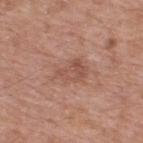Impression:
The lesion was photographed on a routine skin check and not biopsied; there is no pathology result.
Image and clinical context:
A male subject, aged 53–57. An algorithmic analysis of the crop reported a mean CIELAB color near L≈53 a*≈21 b*≈28 and a lesion–skin lightness drop of about 7. The software also gave a border-irregularity index near 4.5/10, a within-lesion color-variation index near 3.5/10, and a peripheral color-asymmetry measure near 1. And it measured a classifier nevus-likeness of about 0/100 and lesion-presence confidence of about 100/100. A 15 mm crop from a total-body photograph taken for skin-cancer surveillance. The recorded lesion diameter is about 4.5 mm. On the upper back. Imaged with white-light lighting.